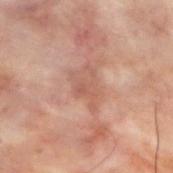Imaged during a routine full-body skin examination; the lesion was not biopsied and no histopathology is available. A close-up tile cropped from a whole-body skin photograph, about 15 mm across. The lesion is on the upper back. A male subject, aged around 30.